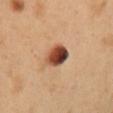  site: chest
  image:
    source: total-body photography crop
    field_of_view_mm: 15
  patient:
    sex: male
    age_approx: 50